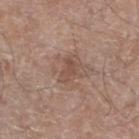Assessment:
This lesion was catalogued during total-body skin photography and was not selected for biopsy.
Background:
Longest diameter approximately 2.5 mm. The tile uses white-light illumination. A 15 mm close-up tile from a total-body photography series done for melanoma screening. A male patient in their 60s. From the left lower leg. The lesion-visualizer software estimated an average lesion color of about L≈49 a*≈18 b*≈26 (CIELAB) and a normalized lesion–skin contrast near 5.5. It also reported a border-irregularity index near 3/10, internal color variation of about 1.5 on a 0–10 scale, and radial color variation of about 0.5. The analysis additionally found an automated nevus-likeness rating near 0 out of 100.